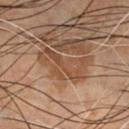Part of a total-body skin-imaging series; this lesion was reviewed on a skin check and was not flagged for biopsy.
Imaged with cross-polarized lighting.
A region of skin cropped from a whole-body photographic capture, roughly 15 mm wide.
The total-body-photography lesion software estimated a lesion area of about 16 mm², an outline eccentricity of about 0.85 (0 = round, 1 = elongated), and two-axis asymmetry of about 0.5. And it measured roughly 7 lightness units darker than nearby skin and a normalized lesion–skin contrast near 6. The analysis additionally found border irregularity of about 6 on a 0–10 scale. And it measured a classifier nevus-likeness of about 25/100 and a detector confidence of about 95 out of 100 that the crop contains a lesion.
A male patient, aged around 60.
The recorded lesion diameter is about 6.5 mm.
On the chest.